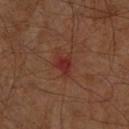Imaged during a routine full-body skin examination; the lesion was not biopsied and no histopathology is available.
A lesion tile, about 15 mm wide, cut from a 3D total-body photograph.
The recorded lesion diameter is about 2.5 mm.
Captured under cross-polarized illumination.
Automated tile analysis of the lesion measured an area of roughly 3.5 mm², an eccentricity of roughly 0.65, and two-axis asymmetry of about 0.4. And it measured a mean CIELAB color near L≈27 a*≈25 b*≈23, a lesion–skin lightness drop of about 7, and a normalized border contrast of about 7.5. It also reported a border-irregularity index near 3.5/10, internal color variation of about 2 on a 0–10 scale, and radial color variation of about 0.5.
A male subject, about 60 years old.
On the right lower leg.